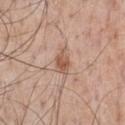Findings:
– workup — total-body-photography surveillance lesion; no biopsy
– imaging modality — total-body-photography crop, ~15 mm field of view
– illumination — white-light
– subject — male, aged approximately 55
– anatomic site — the chest
– automated lesion analysis — internal color variation of about 3 on a 0–10 scale and a peripheral color-asymmetry measure near 1